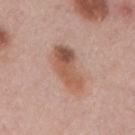Recorded during total-body skin imaging; not selected for excision or biopsy.
The lesion is on the mid back.
A male patient, aged around 55.
Approximately 6 mm at its widest.
A 15 mm crop from a total-body photograph taken for skin-cancer surveillance.